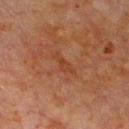{"biopsy_status": "not biopsied; imaged during a skin examination", "image": {"source": "total-body photography crop", "field_of_view_mm": 15}, "site": "chest", "lesion_size": {"long_diameter_mm_approx": 3.0}, "automated_metrics": {"cielab_L": 32, "cielab_a": 20, "cielab_b": 28, "vs_skin_contrast_norm": 5.0, "border_irregularity_0_10": 5.0, "color_variation_0_10": 1.0, "peripheral_color_asymmetry": 0.0}, "lighting": "cross-polarized", "patient": {"sex": "male", "age_approx": 80}}